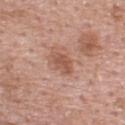No biopsy was performed on this lesion — it was imaged during a full skin examination and was not determined to be concerning. This image is a 15 mm lesion crop taken from a total-body photograph. The patient is a male aged 53 to 57. Imaged with white-light lighting. From the upper back.